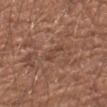Q: Was this lesion biopsied?
A: no biopsy performed (imaged during a skin exam)
Q: How was the tile lit?
A: white-light
Q: Where on the body is the lesion?
A: the left forearm
Q: What is the imaging modality?
A: 15 mm crop, total-body photography
Q: Patient demographics?
A: male, approximately 65 years of age
Q: Lesion size?
A: ≈3 mm
Q: What did automated image analysis measure?
A: two-axis asymmetry of about 0.45; an average lesion color of about L≈43 a*≈21 b*≈27 (CIELAB), roughly 6 lightness units darker than nearby skin, and a lesion-to-skin contrast of about 5 (normalized; higher = more distinct); a border-irregularity rating of about 5/10 and a within-lesion color-variation index near 0/10